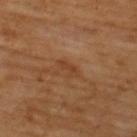follow-up — no biopsy performed (imaged during a skin exam) | location — the upper back | patient — male, aged 63 to 67 | image source — ~15 mm crop, total-body skin-cancer survey | image-analysis metrics — a footprint of about 2.5 mm², an eccentricity of roughly 0.9, and a shape-asymmetry score of about 0.35 (0 = symmetric); a lesion-to-skin contrast of about 5.5 (normalized; higher = more distinct); border irregularity of about 4 on a 0–10 scale, internal color variation of about 0 on a 0–10 scale, and a peripheral color-asymmetry measure near 0.